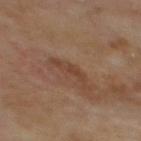Clinical impression:
Captured during whole-body skin photography for melanoma surveillance; the lesion was not biopsied.
Acquisition and patient details:
A male patient, aged approximately 70. The recorded lesion diameter is about 4 mm. A close-up tile cropped from a whole-body skin photograph, about 15 mm across. From the mid back.